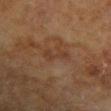Captured during whole-body skin photography for melanoma surveillance; the lesion was not biopsied. The lesion is located on the arm. About 3.5 mm across. This is a cross-polarized tile. Cropped from a total-body skin-imaging series; the visible field is about 15 mm. Automated tile analysis of the lesion measured an area of roughly 3.5 mm² and a shape-asymmetry score of about 0.45 (0 = symmetric). It also reported a lesion color around L≈32 a*≈17 b*≈26 in CIELAB, a lesion–skin lightness drop of about 5, and a normalized border contrast of about 5. It also reported a border-irregularity rating of about 5.5/10, a within-lesion color-variation index near 0.5/10, and peripheral color asymmetry of about 0. The analysis additionally found a lesion-detection confidence of about 100/100. A female subject aged around 80.